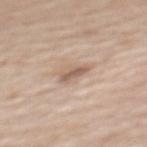Part of a total-body skin-imaging series; this lesion was reviewed on a skin check and was not flagged for biopsy.